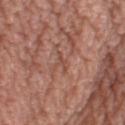Case summary:
- biopsy status — total-body-photography surveillance lesion; no biopsy
- acquisition — ~15 mm tile from a whole-body skin photo
- TBP lesion metrics — an area of roughly 2 mm² and two-axis asymmetry of about 0.4; roughly 7 lightness units darker than nearby skin and a normalized lesion–skin contrast near 5.5; a within-lesion color-variation index near 0/10 and radial color variation of about 0
- lesion diameter — about 2.5 mm
- body site — the leg
- illumination — white-light
- subject — female, roughly 40 years of age